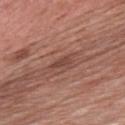No biopsy was performed on this lesion — it was imaged during a full skin examination and was not determined to be concerning. About 3 mm across. The lesion is located on the chest. A male patient in their mid-50s. This image is a 15 mm lesion crop taken from a total-body photograph. Automated image analysis of the tile measured a lesion area of about 3 mm² and an outline eccentricity of about 0.9 (0 = round, 1 = elongated).